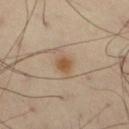Approximately 2.5 mm at its widest. The lesion-visualizer software estimated a lesion color around L≈53 a*≈17 b*≈33 in CIELAB, about 9 CIELAB-L* units darker than the surrounding skin, and a normalized border contrast of about 8. It also reported internal color variation of about 2.5 on a 0–10 scale. The software also gave a nevus-likeness score of about 95/100. The patient is a male aged 53 to 57. The lesion is located on the right thigh. This is a cross-polarized tile. A 15 mm crop from a total-body photograph taken for skin-cancer surveillance.